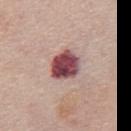Imaged during a routine full-body skin examination; the lesion was not biopsied and no histopathology is available.
The recorded lesion diameter is about 4 mm.
Automated image analysis of the tile measured a lesion area of about 10 mm², a shape eccentricity near 0.5, and a symmetry-axis asymmetry near 0.15. The software also gave a lesion–skin lightness drop of about 20 and a normalized border contrast of about 14.
Cropped from a whole-body photographic skin survey; the tile spans about 15 mm.
Imaged with white-light lighting.
Located on the chest.
The patient is a female aged 68 to 72.